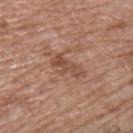This lesion was catalogued during total-body skin photography and was not selected for biopsy. The lesion is on the chest. The patient is a male in their mid- to late 60s. About 4 mm across. A roughly 15 mm field-of-view crop from a total-body skin photograph. This is a white-light tile.